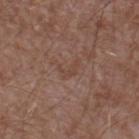Assessment:
The lesion was photographed on a routine skin check and not biopsied; there is no pathology result.
Context:
Imaged with white-light lighting. The patient is a male in their mid- to late 40s. Measured at roughly 3 mm in maximum diameter. Automated tile analysis of the lesion measured a mean CIELAB color near L≈44 a*≈19 b*≈26, about 5 CIELAB-L* units darker than the surrounding skin, and a lesion-to-skin contrast of about 4.5 (normalized; higher = more distinct). The analysis additionally found a border-irregularity rating of about 7.5/10 and internal color variation of about 0 on a 0–10 scale. The analysis additionally found a nevus-likeness score of about 0/100 and a lesion-detection confidence of about 100/100. From the right lower leg. This image is a 15 mm lesion crop taken from a total-body photograph.